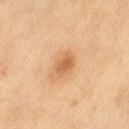Clinical impression: Part of a total-body skin-imaging series; this lesion was reviewed on a skin check and was not flagged for biopsy. Background: A female patient, aged 58 to 62. Approximately 3 mm at its widest. Located on the left thigh. Cropped from a whole-body photographic skin survey; the tile spans about 15 mm.